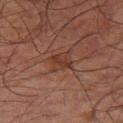Q: Was this lesion biopsied?
A: imaged on a skin check; not biopsied
Q: How was the tile lit?
A: cross-polarized illumination
Q: Patient demographics?
A: male, aged 63 to 67
Q: Where on the body is the lesion?
A: the right thigh
Q: What kind of image is this?
A: total-body-photography crop, ~15 mm field of view